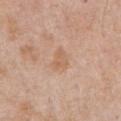The lesion was photographed on a routine skin check and not biopsied; there is no pathology result. Cropped from a whole-body photographic skin survey; the tile spans about 15 mm. The lesion-visualizer software estimated a border-irregularity index near 4/10, a within-lesion color-variation index near 2/10, and radial color variation of about 0.5. A male patient approximately 70 years of age. About 3 mm across. On the abdomen. Captured under white-light illumination.